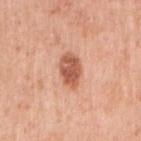No biopsy was performed on this lesion — it was imaged during a full skin examination and was not determined to be concerning. Imaged with white-light lighting. The subject is a female aged 38 to 42. The lesion is on the right upper arm. Approximately 4 mm at its widest. Automated image analysis of the tile measured a lesion area of about 7.5 mm², an outline eccentricity of about 0.8 (0 = round, 1 = elongated), and a shape-asymmetry score of about 0.15 (0 = symmetric). The software also gave a within-lesion color-variation index near 3.5/10 and peripheral color asymmetry of about 1. A 15 mm close-up extracted from a 3D total-body photography capture.